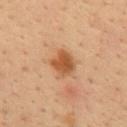Clinical impression: Imaged during a routine full-body skin examination; the lesion was not biopsied and no histopathology is available. Context: This is a cross-polarized tile. A male patient, approximately 35 years of age. The lesion is located on the mid back. A lesion tile, about 15 mm wide, cut from a 3D total-body photograph. The lesion's longest dimension is about 3 mm.